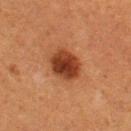notes — imaged on a skin check; not biopsied
image — total-body-photography crop, ~15 mm field of view
patient — female, aged around 40
image-analysis metrics — a border-irregularity rating of about 1.5/10 and a color-variation rating of about 4.5/10
lesion size — ~4 mm (longest diameter)
lighting — cross-polarized
location — the left thigh